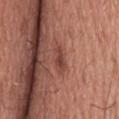Captured during whole-body skin photography for melanoma surveillance; the lesion was not biopsied. The lesion is located on the head or neck. A male patient, aged 73–77. A 15 mm close-up tile from a total-body photography series done for melanoma screening. Imaged with white-light lighting. Automated image analysis of the tile measured a lesion color around L≈44 a*≈25 b*≈26 in CIELAB, about 8 CIELAB-L* units darker than the surrounding skin, and a lesion-to-skin contrast of about 6.5 (normalized; higher = more distinct). Longest diameter approximately 3.5 mm.